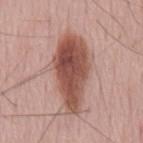Assessment:
The lesion was tiled from a total-body skin photograph and was not biopsied.
Context:
This image is a 15 mm lesion crop taken from a total-body photograph. Longest diameter approximately 8.5 mm. A male patient in their mid-50s. An algorithmic analysis of the crop reported an area of roughly 26 mm², a shape eccentricity near 0.9, and a shape-asymmetry score of about 0.25 (0 = symmetric). It also reported an average lesion color of about L≈51 a*≈23 b*≈26 (CIELAB), about 16 CIELAB-L* units darker than the surrounding skin, and a normalized border contrast of about 10.5. The software also gave a border-irregularity index near 3.5/10, internal color variation of about 6 on a 0–10 scale, and a peripheral color-asymmetry measure near 2.